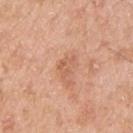Image and clinical context: An algorithmic analysis of the crop reported an eccentricity of roughly 0.75 and a symmetry-axis asymmetry near 0.45. And it measured internal color variation of about 1.5 on a 0–10 scale and radial color variation of about 0.5. The tile uses white-light illumination. The subject is a male approximately 55 years of age. On the left upper arm. Measured at roughly 3 mm in maximum diameter. This image is a 15 mm lesion crop taken from a total-body photograph.